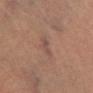Q: What did automated image analysis measure?
A: an area of roughly 2.5 mm², an eccentricity of roughly 0.9, and a symmetry-axis asymmetry near 0.4; border irregularity of about 4 on a 0–10 scale, internal color variation of about 0 on a 0–10 scale, and a peripheral color-asymmetry measure near 0; a nevus-likeness score of about 0/100
Q: Who is the patient?
A: female, aged around 65
Q: How was the tile lit?
A: cross-polarized illumination
Q: What is the imaging modality?
A: total-body-photography crop, ~15 mm field of view
Q: Where on the body is the lesion?
A: the left lower leg
Q: How large is the lesion?
A: ~2.5 mm (longest diameter)